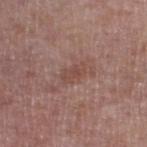Captured during whole-body skin photography for melanoma surveillance; the lesion was not biopsied. A region of skin cropped from a whole-body photographic capture, roughly 15 mm wide. An algorithmic analysis of the crop reported about 7 CIELAB-L* units darker than the surrounding skin and a normalized lesion–skin contrast near 5.5. The software also gave internal color variation of about 2.5 on a 0–10 scale and a peripheral color-asymmetry measure near 1. The lesion is located on the leg. A male subject about 75 years old. This is a white-light tile.